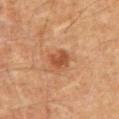The patient is a male in their 60s.
Cropped from a total-body skin-imaging series; the visible field is about 15 mm.
The lesion is on the mid back.
The recorded lesion diameter is about 2.5 mm.
The tile uses cross-polarized illumination.